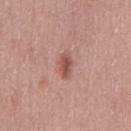biopsy_status: not biopsied; imaged during a skin examination